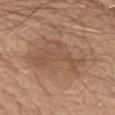notes: total-body-photography surveillance lesion; no biopsy | body site: the front of the torso | lighting: white-light illumination | TBP lesion metrics: a mean CIELAB color near L≈53 a*≈18 b*≈29 and a normalized lesion–skin contrast near 5; a border-irregularity rating of about 4.5/10, a color-variation rating of about 4/10, and radial color variation of about 1.5 | imaging modality: ~15 mm crop, total-body skin-cancer survey | subject: male, in their mid-70s.